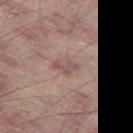No biopsy was performed on this lesion — it was imaged during a full skin examination and was not determined to be concerning.
Captured under white-light illumination.
On the leg.
A roughly 15 mm field-of-view crop from a total-body skin photograph.
Longest diameter approximately 3 mm.
The lesion-visualizer software estimated a footprint of about 4 mm² and a shape eccentricity near 0.85. And it measured roughly 8 lightness units darker than nearby skin and a lesion-to-skin contrast of about 6 (normalized; higher = more distinct). The analysis additionally found a nevus-likeness score of about 0/100 and lesion-presence confidence of about 100/100.
A male subject, aged 63 to 67.